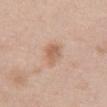Captured during whole-body skin photography for melanoma surveillance; the lesion was not biopsied. From the abdomen. The total-body-photography lesion software estimated an area of roughly 4.5 mm², an eccentricity of roughly 0.8, and two-axis asymmetry of about 0.25. The analysis additionally found a mean CIELAB color near L≈61 a*≈20 b*≈31, a lesion–skin lightness drop of about 9, and a lesion-to-skin contrast of about 6.5 (normalized; higher = more distinct). And it measured a border-irregularity index near 3/10, a color-variation rating of about 2.5/10, and radial color variation of about 1. The analysis additionally found a nevus-likeness score of about 60/100 and a detector confidence of about 100 out of 100 that the crop contains a lesion. A female subject, in their 50s. Longest diameter approximately 3.5 mm. Captured under white-light illumination. A close-up tile cropped from a whole-body skin photograph, about 15 mm across.